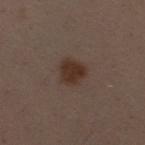Assessment: The lesion was tiled from a total-body skin photograph and was not biopsied. Image and clinical context: The patient is a female aged around 30. Imaged with white-light lighting. Measured at roughly 3 mm in maximum diameter. A close-up tile cropped from a whole-body skin photograph, about 15 mm across. The lesion is located on the right thigh. Automated image analysis of the tile measured a footprint of about 6.5 mm², an outline eccentricity of about 0.5 (0 = round, 1 = elongated), and two-axis asymmetry of about 0.2. The software also gave a lesion color around L≈31 a*≈16 b*≈23 in CIELAB, about 9 CIELAB-L* units darker than the surrounding skin, and a normalized border contrast of about 9.5. The analysis additionally found a peripheral color-asymmetry measure near 0.5.